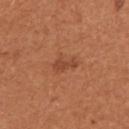Part of a total-body skin-imaging series; this lesion was reviewed on a skin check and was not flagged for biopsy. Measured at roughly 3 mm in maximum diameter. Captured under white-light illumination. The total-body-photography lesion software estimated an eccentricity of roughly 0.9 and a shape-asymmetry score of about 0.35 (0 = symmetric). It also reported a border-irregularity index near 4/10 and a color-variation rating of about 0.5/10. The lesion is located on the arm. A female patient, aged around 55. Cropped from a total-body skin-imaging series; the visible field is about 15 mm.